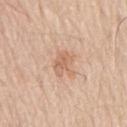<lesion>
<biopsy_status>not biopsied; imaged during a skin examination</biopsy_status>
<patient>
  <sex>male</sex>
  <age_approx>80</age_approx>
</patient>
<lesion_size>
  <long_diameter_mm_approx>3.0</long_diameter_mm_approx>
</lesion_size>
<site>lower back</site>
<image>
  <source>total-body photography crop</source>
  <field_of_view_mm>15</field_of_view_mm>
</image>
<lighting>white-light</lighting>
</lesion>